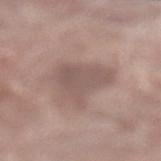Q: Was this lesion biopsied?
A: total-body-photography surveillance lesion; no biopsy
Q: Illumination type?
A: white-light illumination
Q: What are the patient's age and sex?
A: female, roughly 80 years of age
Q: How was this image acquired?
A: total-body-photography crop, ~15 mm field of view
Q: Where on the body is the lesion?
A: the left lower leg
Q: What did automated image analysis measure?
A: an average lesion color of about L≈53 a*≈16 b*≈21 (CIELAB) and a normalized lesion–skin contrast near 6